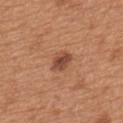Q: Was this lesion biopsied?
A: imaged on a skin check; not biopsied
Q: Automated lesion metrics?
A: a shape eccentricity near 0.75 and a symmetry-axis asymmetry near 0.25; a border-irregularity rating of about 2/10, internal color variation of about 4 on a 0–10 scale, and a peripheral color-asymmetry measure near 1; an automated nevus-likeness rating near 70 out of 100 and lesion-presence confidence of about 100/100
Q: Patient demographics?
A: male, aged 63–67
Q: Lesion size?
A: ~3 mm (longest diameter)
Q: Where on the body is the lesion?
A: the upper back
Q: What is the imaging modality?
A: ~15 mm tile from a whole-body skin photo